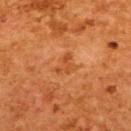Case summary:
* workup — catalogued during a skin exam; not biopsied
* location — the back
* size — ≈3 mm
* TBP lesion metrics — an area of roughly 4.5 mm², an outline eccentricity of about 0.55 (0 = round, 1 = elongated), and a shape-asymmetry score of about 0.45 (0 = symmetric); about 6 CIELAB-L* units darker than the surrounding skin and a lesion-to-skin contrast of about 5 (normalized; higher = more distinct); a nevus-likeness score of about 0/100 and a lesion-detection confidence of about 100/100
* tile lighting — cross-polarized
* subject — female, approximately 50 years of age
* imaging modality — ~15 mm tile from a whole-body skin photo